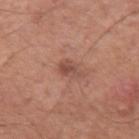Q: Was a biopsy performed?
A: no biopsy performed (imaged during a skin exam)
Q: How was the tile lit?
A: white-light illumination
Q: What is the imaging modality?
A: 15 mm crop, total-body photography
Q: How large is the lesion?
A: ~3 mm (longest diameter)
Q: What is the anatomic site?
A: the left upper arm
Q: Who is the patient?
A: male, in their mid- to late 50s